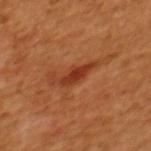The lesion was tiled from a total-body skin photograph and was not biopsied. On the upper back. Imaged with cross-polarized lighting. The patient is a male aged around 45. The lesion's longest dimension is about 4.5 mm. Cropped from a total-body skin-imaging series; the visible field is about 15 mm.